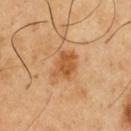Clinical impression:
No biopsy was performed on this lesion — it was imaged during a full skin examination and was not determined to be concerning.
Context:
The lesion is on the front of the torso. A male patient, roughly 50 years of age. A 15 mm crop from a total-body photograph taken for skin-cancer surveillance. Automated tile analysis of the lesion measured a lesion area of about 7.5 mm², a shape eccentricity near 0.7, and two-axis asymmetry of about 0.25. It also reported an average lesion color of about L≈54 a*≈24 b*≈41 (CIELAB), about 10 CIELAB-L* units darker than the surrounding skin, and a lesion-to-skin contrast of about 7.5 (normalized; higher = more distinct). And it measured a lesion-detection confidence of about 100/100. Imaged with cross-polarized lighting.